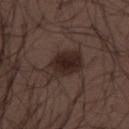Impression: The lesion was photographed on a routine skin check and not biopsied; there is no pathology result. Image and clinical context: A male patient, approximately 30 years of age. A region of skin cropped from a whole-body photographic capture, roughly 15 mm wide. Located on the upper back. The lesion's longest dimension is about 5 mm.